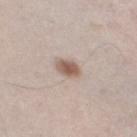workup: imaged on a skin check; not biopsied
image: 15 mm crop, total-body photography
site: the leg
lighting: white-light
subject: male, in their 60s